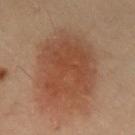diameter: ~9 mm (longest diameter)
location: the chest
image-analysis metrics: a lesion color around L≈36 a*≈18 b*≈26 in CIELAB, a lesion–skin lightness drop of about 7, and a normalized lesion–skin contrast near 7; a border-irregularity rating of about 2.5/10, a within-lesion color-variation index near 3.5/10, and radial color variation of about 1
patient: male, aged 53 to 57
imaging modality: total-body-photography crop, ~15 mm field of view
tile lighting: cross-polarized